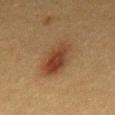Imaged during a routine full-body skin examination; the lesion was not biopsied and no histopathology is available. Imaged with cross-polarized lighting. Longest diameter approximately 6.5 mm. A male subject, about 50 years old. The lesion is located on the front of the torso. Cropped from a total-body skin-imaging series; the visible field is about 15 mm.